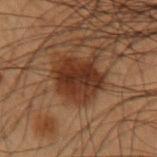Q: Was this lesion biopsied?
A: imaged on a skin check; not biopsied
Q: Automated lesion metrics?
A: a lesion area of about 19 mm², an outline eccentricity of about 0.45 (0 = round, 1 = elongated), and two-axis asymmetry of about 0.3; border irregularity of about 3.5 on a 0–10 scale and peripheral color asymmetry of about 1; an automated nevus-likeness rating near 75 out of 100 and lesion-presence confidence of about 100/100
Q: What is the lesion's diameter?
A: ≈6 mm
Q: What are the patient's age and sex?
A: male, about 55 years old
Q: How was the tile lit?
A: cross-polarized illumination
Q: What is the imaging modality?
A: 15 mm crop, total-body photography
Q: Where on the body is the lesion?
A: the left upper arm